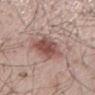Clinical impression:
This lesion was catalogued during total-body skin photography and was not selected for biopsy.
Context:
Located on the mid back. The tile uses white-light illumination. Automated tile analysis of the lesion measured a footprint of about 11 mm² and an eccentricity of roughly 0.5. The software also gave a mean CIELAB color near L≈50 a*≈22 b*≈23, roughly 12 lightness units darker than nearby skin, and a normalized border contrast of about 8.5. The analysis additionally found border irregularity of about 2 on a 0–10 scale, a color-variation rating of about 3.5/10, and a peripheral color-asymmetry measure near 1. The analysis additionally found a nevus-likeness score of about 90/100 and lesion-presence confidence of about 100/100. The subject is a male in their mid- to late 60s. This image is a 15 mm lesion crop taken from a total-body photograph.